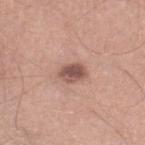  biopsy_status: not biopsied; imaged during a skin examination
  patient:
    sex: male
    age_approx: 55
  lighting: white-light
  site: right lower leg
  lesion_size:
    long_diameter_mm_approx: 3.0
  image:
    source: total-body photography crop
    field_of_view_mm: 15